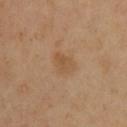No biopsy was performed on this lesion — it was imaged during a full skin examination and was not determined to be concerning.
Imaged with cross-polarized lighting.
On the chest.
A male patient, approximately 40 years of age.
Automated tile analysis of the lesion measured an outline eccentricity of about 0.7 (0 = round, 1 = elongated) and two-axis asymmetry of about 0.25.
Measured at roughly 3 mm in maximum diameter.
A 15 mm close-up tile from a total-body photography series done for melanoma screening.